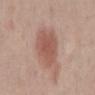Clinical impression:
No biopsy was performed on this lesion — it was imaged during a full skin examination and was not determined to be concerning.
Background:
Located on the mid back. Measured at roughly 5.5 mm in maximum diameter. A male patient, aged 68–72. This image is a 15 mm lesion crop taken from a total-body photograph. Captured under white-light illumination.